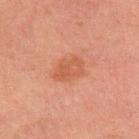Q: Was a biopsy performed?
A: catalogued during a skin exam; not biopsied
Q: What are the patient's age and sex?
A: male, aged 58–62
Q: Lesion size?
A: ~4 mm (longest diameter)
Q: What lighting was used for the tile?
A: cross-polarized
Q: How was this image acquired?
A: total-body-photography crop, ~15 mm field of view
Q: Automated lesion metrics?
A: an area of roughly 9 mm² and two-axis asymmetry of about 0.2; about 6 CIELAB-L* units darker than the surrounding skin; a classifier nevus-likeness of about 20/100 and a lesion-detection confidence of about 100/100
Q: Where on the body is the lesion?
A: the back